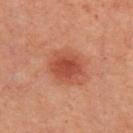Clinical impression:
Captured during whole-body skin photography for melanoma surveillance; the lesion was not biopsied.
Context:
About 4 mm across. A 15 mm close-up tile from a total-body photography series done for melanoma screening. A female subject aged around 45. Automated tile analysis of the lesion measured an eccentricity of roughly 0.5. And it measured a mean CIELAB color near L≈49 a*≈31 b*≈33, roughly 11 lightness units darker than nearby skin, and a normalized border contrast of about 7.5. And it measured border irregularity of about 2 on a 0–10 scale and internal color variation of about 3.5 on a 0–10 scale. The lesion is on the back. Captured under cross-polarized illumination.